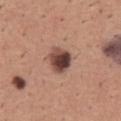This lesion was catalogued during total-body skin photography and was not selected for biopsy. The lesion is on the front of the torso. A male subject, aged 43–47. The lesion's longest dimension is about 3.5 mm. Cropped from a whole-body photographic skin survey; the tile spans about 15 mm. Automated image analysis of the tile measured a mean CIELAB color near L≈44 a*≈21 b*≈23, roughly 18 lightness units darker than nearby skin, and a normalized lesion–skin contrast near 13.